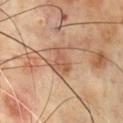biopsy status: catalogued during a skin exam; not biopsied | patient: male, roughly 70 years of age | automated lesion analysis: a lesion color around L≈53 a*≈21 b*≈31 in CIELAB; an automated nevus-likeness rating near 10 out of 100 and a detector confidence of about 100 out of 100 that the crop contains a lesion | tile lighting: cross-polarized | acquisition: ~15 mm crop, total-body skin-cancer survey | site: the front of the torso.